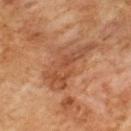  biopsy_status: not biopsied; imaged during a skin examination
  patient:
    sex: male
    age_approx: 60
  image:
    source: total-body photography crop
    field_of_view_mm: 15
  lesion_size:
    long_diameter_mm_approx: 8.0
  site: upper back
  lighting: cross-polarized